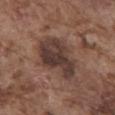Part of a total-body skin-imaging series; this lesion was reviewed on a skin check and was not flagged for biopsy.
Longest diameter approximately 6.5 mm.
This is a white-light tile.
Automated tile analysis of the lesion measured a shape-asymmetry score of about 0.3 (0 = symmetric). The analysis additionally found an average lesion color of about L≈37 a*≈17 b*≈21 (CIELAB) and a normalized lesion–skin contrast near 10. The software also gave border irregularity of about 3.5 on a 0–10 scale, internal color variation of about 4.5 on a 0–10 scale, and radial color variation of about 1.5.
A 15 mm close-up extracted from a 3D total-body photography capture.
The subject is a male about 75 years old.
From the abdomen.